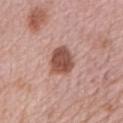Findings:
* workup · no biopsy performed (imaged during a skin exam)
* patient · female, in their mid- to late 60s
* image source · 15 mm crop, total-body photography
* tile lighting · white-light
* lesion size · ~3.5 mm (longest diameter)
* location · the right upper arm